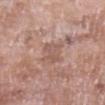Captured during whole-body skin photography for melanoma surveillance; the lesion was not biopsied. A female patient approximately 70 years of age. A close-up tile cropped from a whole-body skin photograph, about 15 mm across. The total-body-photography lesion software estimated border irregularity of about 4 on a 0–10 scale and a within-lesion color-variation index near 3.5/10. The software also gave an automated nevus-likeness rating near 0 out of 100 and lesion-presence confidence of about 100/100. On the left upper arm. Imaged with white-light lighting.